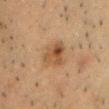Assessment: Imaged during a routine full-body skin examination; the lesion was not biopsied and no histopathology is available. Acquisition and patient details: A male patient roughly 45 years of age. Located on the head or neck. Automated image analysis of the tile measured an area of roughly 8.5 mm² and an eccentricity of roughly 0.6. And it measured a classifier nevus-likeness of about 50/100 and a detector confidence of about 100 out of 100 that the crop contains a lesion. Imaged with cross-polarized lighting. About 3.5 mm across. A 15 mm close-up tile from a total-body photography series done for melanoma screening.